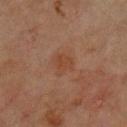Clinical impression: Part of a total-body skin-imaging series; this lesion was reviewed on a skin check and was not flagged for biopsy. Background: Automated tile analysis of the lesion measured a lesion area of about 5 mm², an eccentricity of roughly 0.45, and a symmetry-axis asymmetry near 0.4. It also reported a within-lesion color-variation index near 1.5/10 and peripheral color asymmetry of about 0.5. The analysis additionally found a nevus-likeness score of about 10/100. A male subject, aged 63–67. About 2.5 mm across. A region of skin cropped from a whole-body photographic capture, roughly 15 mm wide. Located on the upper back.